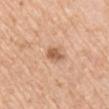The patient is a male aged around 60. Located on the left upper arm. Longest diameter approximately 2.5 mm. This is a white-light tile. A roughly 15 mm field-of-view crop from a total-body skin photograph.